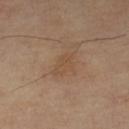workup=no biopsy performed (imaged during a skin exam)
subject=female, in their 70s
site=the left lower leg
automated lesion analysis=a nevus-likeness score of about 0/100 and lesion-presence confidence of about 100/100
acquisition=~15 mm tile from a whole-body skin photo
size=≈3.5 mm